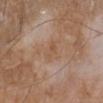Part of a total-body skin-imaging series; this lesion was reviewed on a skin check and was not flagged for biopsy.
Automated tile analysis of the lesion measured an outline eccentricity of about 0.75 (0 = round, 1 = elongated) and two-axis asymmetry of about 0.35. The software also gave a mean CIELAB color near L≈53 a*≈18 b*≈31, a lesion–skin lightness drop of about 5, and a normalized border contrast of about 5. And it measured a color-variation rating of about 3/10. The software also gave lesion-presence confidence of about 100/100.
A male subject, approximately 65 years of age.
This image is a 15 mm lesion crop taken from a total-body photograph.
The tile uses white-light illumination.
Located on the left lower leg.